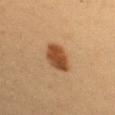The lesion was photographed on a routine skin check and not biopsied; there is no pathology result. Imaged with cross-polarized lighting. A female patient approximately 40 years of age. A roughly 15 mm field-of-view crop from a total-body skin photograph. On the left upper arm. The total-body-photography lesion software estimated an area of roughly 9 mm², a shape eccentricity near 0.65, and a symmetry-axis asymmetry near 0.2. The analysis additionally found border irregularity of about 2 on a 0–10 scale and radial color variation of about 1. The analysis additionally found a classifier nevus-likeness of about 100/100 and lesion-presence confidence of about 100/100. The lesion's longest dimension is about 4 mm.